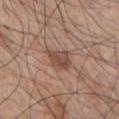body site: the chest; tile lighting: white-light illumination; image: total-body-photography crop, ~15 mm field of view; patient: male, roughly 70 years of age; lesion size: ~3 mm (longest diameter).